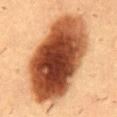The lesion was tiled from a total-body skin photograph and was not biopsied. Cropped from a total-body skin-imaging series; the visible field is about 15 mm. On the mid back. A male subject aged around 55. Approximately 12 mm at its widest. Captured under cross-polarized illumination.